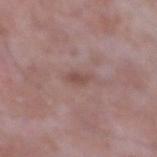Recorded during total-body skin imaging; not selected for excision or biopsy. A male patient, about 65 years old. The lesion is located on the left forearm. A close-up tile cropped from a whole-body skin photograph, about 15 mm across.